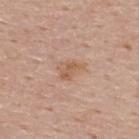Findings:
• follow-up: catalogued during a skin exam; not biopsied
• patient: male, about 55 years old
• acquisition: total-body-photography crop, ~15 mm field of view
• location: the upper back
• lighting: white-light
• size: ≈3 mm